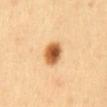Part of a total-body skin-imaging series; this lesion was reviewed on a skin check and was not flagged for biopsy.
Longest diameter approximately 3.5 mm.
A female subject, aged 48–52.
Imaged with cross-polarized lighting.
From the front of the torso.
A lesion tile, about 15 mm wide, cut from a 3D total-body photograph.